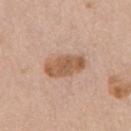Q: Was this lesion biopsied?
A: no biopsy performed (imaged during a skin exam)
Q: What did automated image analysis measure?
A: a shape eccentricity near 0.85 and a symmetry-axis asymmetry near 0.2; a lesion color around L≈57 a*≈20 b*≈32 in CIELAB and a lesion–skin lightness drop of about 11; a classifier nevus-likeness of about 30/100 and a detector confidence of about 100 out of 100 that the crop contains a lesion
Q: How was the tile lit?
A: white-light
Q: How was this image acquired?
A: ~15 mm tile from a whole-body skin photo
Q: What are the patient's age and sex?
A: female, roughly 70 years of age
Q: What is the lesion's diameter?
A: ≈5 mm
Q: What is the anatomic site?
A: the chest